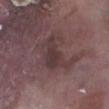Q: Was a biopsy performed?
A: catalogued during a skin exam; not biopsied
Q: How large is the lesion?
A: ~5.5 mm (longest diameter)
Q: What is the imaging modality?
A: ~15 mm crop, total-body skin-cancer survey
Q: Automated lesion metrics?
A: a lesion area of about 14 mm², an outline eccentricity of about 0.75 (0 = round, 1 = elongated), and a shape-asymmetry score of about 0.5 (0 = symmetric); an average lesion color of about L≈36 a*≈17 b*≈15 (CIELAB) and a normalized lesion–skin contrast near 6.5
Q: What are the patient's age and sex?
A: male, roughly 75 years of age
Q: Where on the body is the lesion?
A: the right lower leg